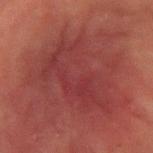Captured during whole-body skin photography for melanoma surveillance; the lesion was not biopsied. On the arm. A male subject, in their 70s. Captured under cross-polarized illumination. A 15 mm close-up extracted from a 3D total-body photography capture. The recorded lesion diameter is about 10 mm. Automated tile analysis of the lesion measured an area of roughly 50 mm² and two-axis asymmetry of about 0.3. It also reported a mean CIELAB color near L≈32 a*≈26 b*≈20 and roughly 5 lightness units darker than nearby skin. It also reported a nevus-likeness score of about 0/100 and lesion-presence confidence of about 85/100.